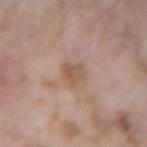A 15 mm close-up extracted from a 3D total-body photography capture. A male patient, aged around 60. On the arm.